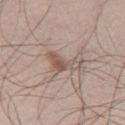{"biopsy_status": "not biopsied; imaged during a skin examination", "automated_metrics": {"border_irregularity_0_10": 6.5, "color_variation_0_10": 5.5, "peripheral_color_asymmetry": 1.5, "nevus_likeness_0_100": 25, "lesion_detection_confidence_0_100": 100}, "site": "right thigh", "lighting": "white-light", "patient": {"sex": "male", "age_approx": 50}, "image": {"source": "total-body photography crop", "field_of_view_mm": 15}}